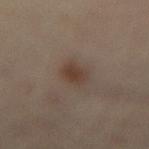The lesion is located on the lower back.
The subject is a female in their 60s.
Cropped from a total-body skin-imaging series; the visible field is about 15 mm.
Measured at roughly 2.5 mm in maximum diameter.
The total-body-photography lesion software estimated a lesion-to-skin contrast of about 7.5 (normalized; higher = more distinct). The software also gave a border-irregularity rating of about 2/10, internal color variation of about 2 on a 0–10 scale, and peripheral color asymmetry of about 0.5. The analysis additionally found lesion-presence confidence of about 100/100.
The tile uses cross-polarized illumination.